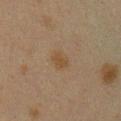Impression: Captured during whole-body skin photography for melanoma surveillance; the lesion was not biopsied. Background: Longest diameter approximately 2.5 mm. Located on the chest. Cropped from a whole-body photographic skin survey; the tile spans about 15 mm. A female patient aged 38–42. Imaged with cross-polarized lighting.